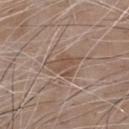Imaged during a routine full-body skin examination; the lesion was not biopsied and no histopathology is available. A close-up tile cropped from a whole-body skin photograph, about 15 mm across. Approximately 3.5 mm at its widest. From the chest. The total-body-photography lesion software estimated a mean CIELAB color near L≈50 a*≈17 b*≈26, about 8 CIELAB-L* units darker than the surrounding skin, and a normalized lesion–skin contrast near 6. The software also gave a peripheral color-asymmetry measure near 0.5. The software also gave an automated nevus-likeness rating near 0 out of 100 and a lesion-detection confidence of about 100/100. A male subject in their 80s. Imaged with white-light lighting.